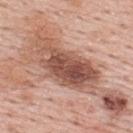workup: imaged on a skin check; not biopsied | lesion diameter: about 8.5 mm | imaging modality: ~15 mm tile from a whole-body skin photo | patient: male, aged 53–57 | body site: the upper back | tile lighting: white-light illumination.